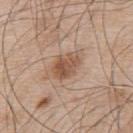Recorded during total-body skin imaging; not selected for excision or biopsy.
The tile uses white-light illumination.
A male patient, approximately 55 years of age.
A 15 mm close-up tile from a total-body photography series done for melanoma screening.
About 4 mm across.
From the upper back.
Automated tile analysis of the lesion measured a footprint of about 8.5 mm², an eccentricity of roughly 0.75, and two-axis asymmetry of about 0.15.